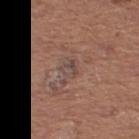No biopsy was performed on this lesion — it was imaged during a full skin examination and was not determined to be concerning. A female patient aged 48–52. Automated image analysis of the tile measured roughly 6 lightness units darker than nearby skin and a normalized lesion–skin contrast near 5.5. A lesion tile, about 15 mm wide, cut from a 3D total-body photograph. The tile uses white-light illumination. The lesion's longest dimension is about 3.5 mm. The lesion is located on the right thigh.